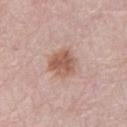This lesion was catalogued during total-body skin photography and was not selected for biopsy.
The subject is a male aged 78–82.
The lesion is located on the left lower leg.
This is a white-light tile.
Cropped from a total-body skin-imaging series; the visible field is about 15 mm.
Measured at roughly 4 mm in maximum diameter.